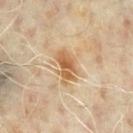Impression:
The lesion was photographed on a routine skin check and not biopsied; there is no pathology result.
Image and clinical context:
Approximately 4.5 mm at its widest. A male patient aged approximately 65. On the chest. A lesion tile, about 15 mm wide, cut from a 3D total-body photograph.